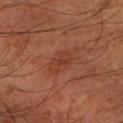The lesion was tiled from a total-body skin photograph and was not biopsied. The lesion is located on the left forearm. The recorded lesion diameter is about 3.5 mm. A male subject, aged 58 to 62. A close-up tile cropped from a whole-body skin photograph, about 15 mm across. The tile uses cross-polarized illumination.